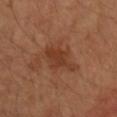This lesion was catalogued during total-body skin photography and was not selected for biopsy.
The lesion is on the right forearm.
Automated tile analysis of the lesion measured a nevus-likeness score of about 5/100 and a lesion-detection confidence of about 100/100.
A female patient aged approximately 60.
Captured under cross-polarized illumination.
Longest diameter approximately 4.5 mm.
A 15 mm close-up tile from a total-body photography series done for melanoma screening.